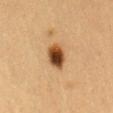From the mid back.
A 15 mm close-up extracted from a 3D total-body photography capture.
A female patient aged 38 to 42.